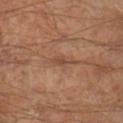{"biopsy_status": "not biopsied; imaged during a skin examination", "automated_metrics": {"lesion_detection_confidence_0_100": 55}, "lighting": "cross-polarized", "patient": {"sex": "male", "age_approx": 65}, "image": {"source": "total-body photography crop", "field_of_view_mm": 15}, "site": "right lower leg"}